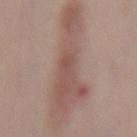Clinical impression: Recorded during total-body skin imaging; not selected for excision or biopsy. Acquisition and patient details: A lesion tile, about 15 mm wide, cut from a 3D total-body photograph. The subject is a female aged approximately 65. The lesion is on the mid back. The total-body-photography lesion software estimated an area of roughly 38 mm², an outline eccentricity of about 0.95 (0 = round, 1 = elongated), and a symmetry-axis asymmetry near 0.4. The software also gave an average lesion color of about L≈53 a*≈18 b*≈23 (CIELAB), roughly 9 lightness units darker than nearby skin, and a lesion-to-skin contrast of about 6 (normalized; higher = more distinct). The software also gave a border-irregularity index near 6/10, a within-lesion color-variation index near 4.5/10, and radial color variation of about 1.5. The software also gave a nevus-likeness score of about 0/100 and lesion-presence confidence of about 90/100. This is a white-light tile.